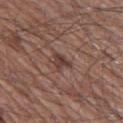notes — catalogued during a skin exam; not biopsied | lighting — white-light illumination | patient — male, about 60 years old | acquisition — 15 mm crop, total-body photography | body site — the left thigh.